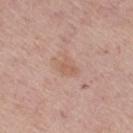Findings:
– imaging modality: total-body-photography crop, ~15 mm field of view
– location: the right thigh
– lighting: white-light
– automated lesion analysis: a border-irregularity index near 3.5/10, a within-lesion color-variation index near 1.5/10, and peripheral color asymmetry of about 0.5; an automated nevus-likeness rating near 0 out of 100 and a lesion-detection confidence of about 100/100
– diameter: ≈3.5 mm
– patient: female, aged 63–67